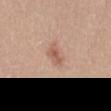No biopsy was performed on this lesion — it was imaged during a full skin examination and was not determined to be concerning.
A female patient, roughly 35 years of age.
About 3 mm across.
From the mid back.
A 15 mm crop from a total-body photograph taken for skin-cancer surveillance.